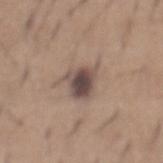Q: Was a biopsy performed?
A: imaged on a skin check; not biopsied
Q: What kind of image is this?
A: ~15 mm tile from a whole-body skin photo
Q: Where on the body is the lesion?
A: the mid back
Q: Who is the patient?
A: male, roughly 65 years of age